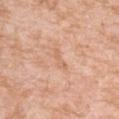Recorded during total-body skin imaging; not selected for excision or biopsy. The patient is a female aged 38–42. Automated tile analysis of the lesion measured two-axis asymmetry of about 0.45. The software also gave an automated nevus-likeness rating near 0 out of 100 and a lesion-detection confidence of about 100/100. About 3 mm across. This is a white-light tile. From the left forearm. This image is a 15 mm lesion crop taken from a total-body photograph.